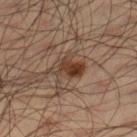The lesion was tiled from a total-body skin photograph and was not biopsied. A region of skin cropped from a whole-body photographic capture, roughly 15 mm wide. A male subject, about 35 years old. Located on the right lower leg.